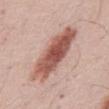{"automated_metrics": {"area_mm2_approx": 21.0, "eccentricity": 0.9, "shape_asymmetry": 0.2, "border_irregularity_0_10": 3.0, "color_variation_0_10": 5.5}, "patient": {"sex": "male", "age_approx": 55}, "lighting": "white-light", "site": "mid back", "image": {"source": "total-body photography crop", "field_of_view_mm": 15}, "lesion_size": {"long_diameter_mm_approx": 8.0}}